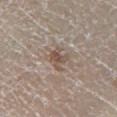<tbp_lesion>
  <lighting>white-light</lighting>
  <site>right lower leg</site>
  <lesion_size>
    <long_diameter_mm_approx>3.0</long_diameter_mm_approx>
  </lesion_size>
  <patient>
    <sex>male</sex>
    <age_approx>60</age_approx>
  </patient>
  <image>
    <source>total-body photography crop</source>
    <field_of_view_mm>15</field_of_view_mm>
  </image>
  <automated_metrics>
    <area_mm2_approx>4.5</area_mm2_approx>
    <eccentricity>0.6</eccentricity>
    <shape_asymmetry>0.35</shape_asymmetry>
  </automated_metrics>
</tbp_lesion>